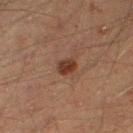notes: imaged on a skin check; not biopsied
size: ≈2.5 mm
site: the right thigh
imaging modality: total-body-photography crop, ~15 mm field of view
automated lesion analysis: a lesion area of about 3.5 mm², an eccentricity of roughly 0.75, and a shape-asymmetry score of about 0.25 (0 = symmetric); an average lesion color of about L≈29 a*≈19 b*≈24 (CIELAB), a lesion–skin lightness drop of about 10, and a normalized lesion–skin contrast near 10
tile lighting: cross-polarized illumination
patient: male, roughly 45 years of age